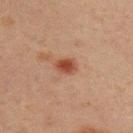notes=no biopsy performed (imaged during a skin exam).